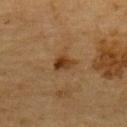Captured during whole-body skin photography for melanoma surveillance; the lesion was not biopsied.
Imaged with cross-polarized lighting.
A male subject, roughly 85 years of age or older.
Automated image analysis of the tile measured an area of roughly 4 mm². It also reported a border-irregularity index near 3/10 and internal color variation of about 5.5 on a 0–10 scale. It also reported an automated nevus-likeness rating near 95 out of 100.
The lesion is on the upper back.
Longest diameter approximately 3 mm.
Cropped from a whole-body photographic skin survey; the tile spans about 15 mm.